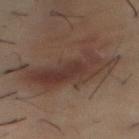Impression: The lesion was tiled from a total-body skin photograph and was not biopsied. Acquisition and patient details: The total-body-photography lesion software estimated a lesion color around L≈28 a*≈14 b*≈18 in CIELAB, about 7 CIELAB-L* units darker than the surrounding skin, and a normalized border contrast of about 7.5. The analysis additionally found a border-irregularity index near 7.5/10 and a color-variation rating of about 4/10. The analysis additionally found a classifier nevus-likeness of about 5/100 and a lesion-detection confidence of about 100/100. This is a cross-polarized tile. A close-up tile cropped from a whole-body skin photograph, about 15 mm across. The lesion is located on the front of the torso. A male patient aged 28 to 32.